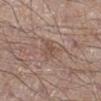Part of a total-body skin-imaging series; this lesion was reviewed on a skin check and was not flagged for biopsy. The lesion is located on the right lower leg. A close-up tile cropped from a whole-body skin photograph, about 15 mm across. This is a white-light tile. Longest diameter approximately 3 mm. Automated tile analysis of the lesion measured a footprint of about 5 mm², an eccentricity of roughly 0.75, and a symmetry-axis asymmetry near 0.4. And it measured a lesion color around L≈51 a*≈17 b*≈25 in CIELAB and about 7 CIELAB-L* units darker than the surrounding skin. It also reported a border-irregularity index near 4/10 and internal color variation of about 1.5 on a 0–10 scale. The analysis additionally found a classifier nevus-likeness of about 0/100 and a detector confidence of about 100 out of 100 that the crop contains a lesion. A male patient aged 53–57.